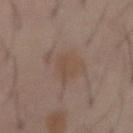The lesion was tiled from a total-body skin photograph and was not biopsied. A male patient, aged 58–62. On the abdomen. Cropped from a total-body skin-imaging series; the visible field is about 15 mm.